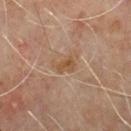| field | value |
|---|---|
| biopsy status | no biopsy performed (imaged during a skin exam) |
| lesion size | ≈2.5 mm |
| patient | male, roughly 60 years of age |
| site | the back |
| illumination | cross-polarized |
| TBP lesion metrics | an average lesion color of about L≈49 a*≈18 b*≈32 (CIELAB) and a lesion–skin lightness drop of about 7 |
| image | total-body-photography crop, ~15 mm field of view |